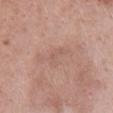<record>
<site>front of the torso</site>
<patient>
  <sex>female</sex>
  <age_approx>45</age_approx>
</patient>
<lesion_size>
  <long_diameter_mm_approx>3.0</long_diameter_mm_approx>
</lesion_size>
<lighting>white-light</lighting>
<image>
  <source>total-body photography crop</source>
  <field_of_view_mm>15</field_of_view_mm>
</image>
</record>